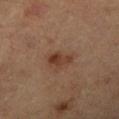Recorded during total-body skin imaging; not selected for excision or biopsy.
A male subject, approximately 85 years of age or older.
A 15 mm close-up tile from a total-body photography series done for melanoma screening.
Longest diameter approximately 3 mm.
The lesion is located on the right lower leg.
The tile uses cross-polarized illumination.